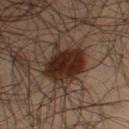Recorded during total-body skin imaging; not selected for excision or biopsy. Automated image analysis of the tile measured an average lesion color of about L≈24 a*≈16 b*≈22 (CIELAB), about 13 CIELAB-L* units darker than the surrounding skin, and a normalized border contrast of about 13.5. It also reported a border-irregularity rating of about 3.5/10, internal color variation of about 4.5 on a 0–10 scale, and peripheral color asymmetry of about 1.5. A 15 mm crop from a total-body photograph taken for skin-cancer surveillance. Imaged with cross-polarized lighting. The patient is a male aged approximately 35. From the left thigh.